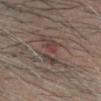Assessment:
Recorded during total-body skin imaging; not selected for excision or biopsy.
Acquisition and patient details:
The lesion's longest dimension is about 6.5 mm. Imaged with cross-polarized lighting. The lesion is on the head or neck. Automated image analysis of the tile measured internal color variation of about 7 on a 0–10 scale and peripheral color asymmetry of about 2.5. The subject is a male in their mid-60s. A region of skin cropped from a whole-body photographic capture, roughly 15 mm wide.